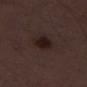Impression: No biopsy was performed on this lesion — it was imaged during a full skin examination and was not determined to be concerning. Image and clinical context: Automated image analysis of the tile measured a lesion area of about 5.5 mm², an outline eccentricity of about 0.6 (0 = round, 1 = elongated), and two-axis asymmetry of about 0.2. The software also gave a border-irregularity index near 1.5/10, internal color variation of about 2.5 on a 0–10 scale, and a peripheral color-asymmetry measure near 1. It also reported a classifier nevus-likeness of about 80/100 and lesion-presence confidence of about 100/100. Captured under white-light illumination. A lesion tile, about 15 mm wide, cut from a 3D total-body photograph. A male subject about 50 years old. The recorded lesion diameter is about 3 mm.